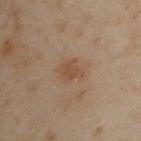notes — imaged on a skin check; not biopsied | patient — male, aged around 50 | tile lighting — cross-polarized illumination | location — the left arm | image — ~15 mm tile from a whole-body skin photo.